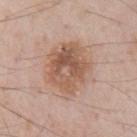follow-up = catalogued during a skin exam; not biopsied | body site = the chest | lighting = white-light | image = ~15 mm tile from a whole-body skin photo | automated lesion analysis = a lesion color around L≈56 a*≈19 b*≈29 in CIELAB and a lesion–skin lightness drop of about 11; an automated nevus-likeness rating near 35 out of 100 and a lesion-detection confidence of about 100/100 | size = ~6 mm (longest diameter) | patient = male, aged 58 to 62.